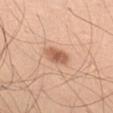notes: no biopsy performed (imaged during a skin exam) | body site: the front of the torso | subject: male, roughly 50 years of age | image: total-body-photography crop, ~15 mm field of view.